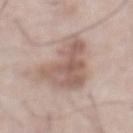Imaged during a routine full-body skin examination; the lesion was not biopsied and no histopathology is available.
The tile uses white-light illumination.
A male subject aged approximately 75.
From the abdomen.
An algorithmic analysis of the crop reported a footprint of about 25 mm², an outline eccentricity of about 0.85 (0 = round, 1 = elongated), and a symmetry-axis asymmetry near 0.5. And it measured roughly 11 lightness units darker than nearby skin and a normalized border contrast of about 7.5.
A region of skin cropped from a whole-body photographic capture, roughly 15 mm wide.
Measured at roughly 8.5 mm in maximum diameter.